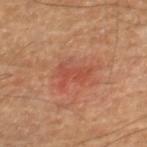<lesion>
  <biopsy_status>not biopsied; imaged during a skin examination</biopsy_status>
  <site>right lower leg</site>
  <patient>
    <sex>male</sex>
    <age_approx>55</age_approx>
  </patient>
  <lighting>cross-polarized</lighting>
  <image>
    <source>total-body photography crop</source>
    <field_of_view_mm>15</field_of_view_mm>
  </image>
  <automated_metrics>
    <vs_skin_contrast_norm>5.0</vs_skin_contrast_norm>
    <border_irregularity_0_10>6.5</border_irregularity_0_10>
    <color_variation_0_10>2.0</color_variation_0_10>
    <peripheral_color_asymmetry>0.5</peripheral_color_asymmetry>
    <nevus_likeness_0_100>5</nevus_likeness_0_100>
  </automated_metrics>
</lesion>